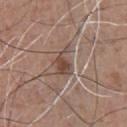Part of a total-body skin-imaging series; this lesion was reviewed on a skin check and was not flagged for biopsy.
A male subject, aged 53 to 57.
Located on the chest.
The lesion's longest dimension is about 4 mm.
Captured under white-light illumination.
A lesion tile, about 15 mm wide, cut from a 3D total-body photograph.